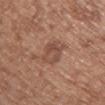Impression: No biopsy was performed on this lesion — it was imaged during a full skin examination and was not determined to be concerning. Background: The lesion is on the chest. A female subject, approximately 75 years of age. Captured under white-light illumination. A roughly 15 mm field-of-view crop from a total-body skin photograph. The recorded lesion diameter is about 3 mm.